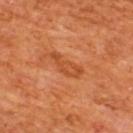biopsy_status: not biopsied; imaged during a skin examination
lesion_size:
  long_diameter_mm_approx: 4.0
site: upper back
lighting: cross-polarized
automated_metrics:
  area_mm2_approx: 4.5
  eccentricity: 0.95
  shape_asymmetry: 0.45
  cielab_L: 48
  cielab_a: 30
  cielab_b: 41
  vs_skin_darker_L: 7.0
  vs_skin_contrast_norm: 6.0
  border_irregularity_0_10: 6.0
  color_variation_0_10: 1.0
  peripheral_color_asymmetry: 0.0
  nevus_likeness_0_100: 0
  lesion_detection_confidence_0_100: 100
patient:
  sex: male
  age_approx: 65
image:
  source: total-body photography crop
  field_of_view_mm: 15